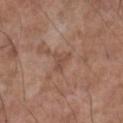<record>
  <biopsy_status>not biopsied; imaged during a skin examination</biopsy_status>
  <site>right lower leg</site>
  <image>
    <source>total-body photography crop</source>
    <field_of_view_mm>15</field_of_view_mm>
  </image>
  <lesion_size>
    <long_diameter_mm_approx>2.5</long_diameter_mm_approx>
  </lesion_size>
  <patient>
    <sex>male</sex>
    <age_approx>55</age_approx>
  </patient>
  <lighting>white-light</lighting>
</record>